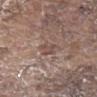Part of a total-body skin-imaging series; this lesion was reviewed on a skin check and was not flagged for biopsy. The lesion's longest dimension is about 2.5 mm. A 15 mm close-up extracted from a 3D total-body photography capture. Imaged with white-light lighting. The patient is a male about 80 years old. The lesion is on the back.